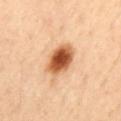Clinical impression: Imaged during a routine full-body skin examination; the lesion was not biopsied and no histopathology is available. Background: Located on the upper back. A 15 mm crop from a total-body photograph taken for skin-cancer surveillance. A male subject, aged 53–57.